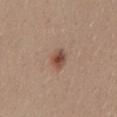Clinical impression: Recorded during total-body skin imaging; not selected for excision or biopsy. Context: A lesion tile, about 15 mm wide, cut from a 3D total-body photograph. Located on the mid back. The patient is a female aged 38–42.